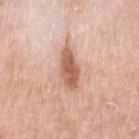Captured during whole-body skin photography for melanoma surveillance; the lesion was not biopsied.
Located on the right upper arm.
A female subject, aged 63 to 67.
Automated image analysis of the tile measured a footprint of about 9.5 mm², an outline eccentricity of about 0.9 (0 = round, 1 = elongated), and a shape-asymmetry score of about 0.2 (0 = symmetric). And it measured a lesion color around L≈61 a*≈23 b*≈31 in CIELAB, about 14 CIELAB-L* units darker than the surrounding skin, and a lesion-to-skin contrast of about 8.5 (normalized; higher = more distinct). And it measured border irregularity of about 2.5 on a 0–10 scale, internal color variation of about 3.5 on a 0–10 scale, and peripheral color asymmetry of about 1.
This image is a 15 mm lesion crop taken from a total-body photograph.